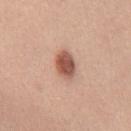Imaged during a routine full-body skin examination; the lesion was not biopsied and no histopathology is available. About 3.5 mm across. Located on the chest. The patient is a female about 40 years old. Captured under white-light illumination. Automated tile analysis of the lesion measured a shape eccentricity near 0.75 and a symmetry-axis asymmetry near 0.1. It also reported a mean CIELAB color near L≈54 a*≈24 b*≈29, roughly 16 lightness units darker than nearby skin, and a normalized lesion–skin contrast near 10. The analysis additionally found an automated nevus-likeness rating near 100 out of 100 and lesion-presence confidence of about 100/100. This image is a 15 mm lesion crop taken from a total-body photograph.